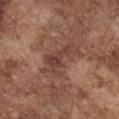Notes:
- biopsy status — no biopsy performed (imaged during a skin exam)
- anatomic site — the chest
- patient — male, aged 73 to 77
- acquisition — 15 mm crop, total-body photography
- TBP lesion metrics — a lesion area of about 10 mm², an outline eccentricity of about 0.85 (0 = round, 1 = elongated), and a symmetry-axis asymmetry near 0.55; a lesion color around L≈42 a*≈20 b*≈25 in CIELAB; border irregularity of about 7.5 on a 0–10 scale and a peripheral color-asymmetry measure near 1; a nevus-likeness score of about 0/100 and a detector confidence of about 90 out of 100 that the crop contains a lesion
- diameter — ~5.5 mm (longest diameter)
- tile lighting — white-light illumination